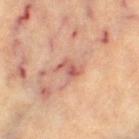Notes:
- follow-up: no biopsy performed (imaged during a skin exam)
- site: the leg
- image source: ~15 mm tile from a whole-body skin photo
- patient: female, roughly 65 years of age
- size: ~2.5 mm (longest diameter)
- illumination: cross-polarized